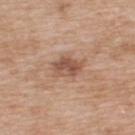Q: Was this lesion biopsied?
A: no biopsy performed (imaged during a skin exam)
Q: What kind of image is this?
A: 15 mm crop, total-body photography
Q: What are the patient's age and sex?
A: male, roughly 50 years of age
Q: What did automated image analysis measure?
A: a border-irregularity rating of about 2.5/10, internal color variation of about 4.5 on a 0–10 scale, and peripheral color asymmetry of about 2; an automated nevus-likeness rating near 10 out of 100 and a detector confidence of about 100 out of 100 that the crop contains a lesion
Q: What lighting was used for the tile?
A: white-light
Q: Lesion location?
A: the upper back